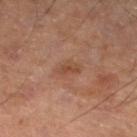biopsy status = total-body-photography surveillance lesion; no biopsy | site = the left lower leg | subject = male, aged around 55 | acquisition = 15 mm crop, total-body photography.